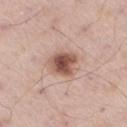Part of a total-body skin-imaging series; this lesion was reviewed on a skin check and was not flagged for biopsy. A male subject, aged 53–57. Measured at roughly 3.5 mm in maximum diameter. A roughly 15 mm field-of-view crop from a total-body skin photograph. The lesion is located on the right thigh.